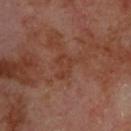biopsy_status: not biopsied; imaged during a skin examination
patient:
  sex: male
  age_approx: 70
lesion_size:
  long_diameter_mm_approx: 4.0
site: upper back
lighting: cross-polarized
image:
  source: total-body photography crop
  field_of_view_mm: 15
automated_metrics:
  cielab_L: 37
  cielab_a: 22
  cielab_b: 28
  vs_skin_darker_L: 5.0
  vs_skin_contrast_norm: 5.0
  border_irregularity_0_10: 6.0
  color_variation_0_10: 2.0
  peripheral_color_asymmetry: 0.5